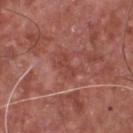workup: no biopsy performed (imaged during a skin exam) | TBP lesion metrics: an area of roughly 4.5 mm² and a shape-asymmetry score of about 0.35 (0 = symmetric); a classifier nevus-likeness of about 0/100 | subject: male, aged 63 to 67 | size: about 3 mm | image: total-body-photography crop, ~15 mm field of view | body site: the chest.